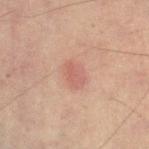diameter = about 3.5 mm | subject = male, aged approximately 50 | imaging modality = 15 mm crop, total-body photography | body site = the right lower leg | lighting = cross-polarized.